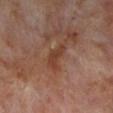Impression:
The lesion was tiled from a total-body skin photograph and was not biopsied.
Background:
Cropped from a whole-body photographic skin survey; the tile spans about 15 mm. The subject is a male about 70 years old. The tile uses cross-polarized illumination. From the left lower leg. About 3.5 mm across.